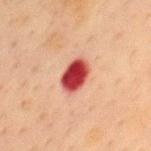Imaged during a routine full-body skin examination; the lesion was not biopsied and no histopathology is available. Automated image analysis of the tile measured a border-irregularity index near 1.5/10, internal color variation of about 4.5 on a 0–10 scale, and peripheral color asymmetry of about 1.5. A 15 mm crop from a total-body photograph taken for skin-cancer surveillance. This is a cross-polarized tile. The recorded lesion diameter is about 3.5 mm. The lesion is on the chest. The patient is a female in their mid-50s.